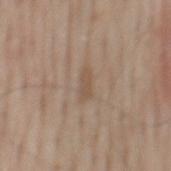Case summary:
- biopsy status: total-body-photography surveillance lesion; no biopsy
- lesion diameter: ≈3 mm
- body site: the mid back
- subject: male, about 55 years old
- tile lighting: white-light illumination
- acquisition: total-body-photography crop, ~15 mm field of view
- automated metrics: a footprint of about 3.5 mm², an eccentricity of roughly 0.9, and two-axis asymmetry of about 0.3; a border-irregularity index near 3.5/10, a within-lesion color-variation index near 0.5/10, and radial color variation of about 0; an automated nevus-likeness rating near 0 out of 100 and a detector confidence of about 100 out of 100 that the crop contains a lesion